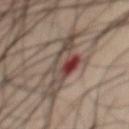Imaged during a routine full-body skin examination; the lesion was not biopsied and no histopathology is available.
A 15 mm close-up extracted from a 3D total-body photography capture.
An algorithmic analysis of the crop reported a lesion–skin lightness drop of about 11. The analysis additionally found an automated nevus-likeness rating near 0 out of 100 and a detector confidence of about 100 out of 100 that the crop contains a lesion.
Approximately 8 mm at its widest.
The patient is a male aged approximately 50.
The lesion is on the chest.
The tile uses cross-polarized illumination.